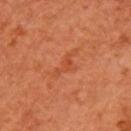The lesion was tiled from a total-body skin photograph and was not biopsied.
Captured under cross-polarized illumination.
A female subject, aged approximately 65.
The lesion is on the right upper arm.
A 15 mm close-up extracted from a 3D total-body photography capture.
Approximately 3 mm at its widest.